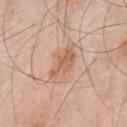follow-up: imaged on a skin check; not biopsied
patient: male, roughly 55 years of age
image: 15 mm crop, total-body photography
tile lighting: white-light illumination
body site: the chest